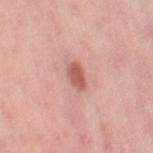Impression:
No biopsy was performed on this lesion — it was imaged during a full skin examination and was not determined to be concerning.
Context:
The lesion-visualizer software estimated a lesion area of about 4.5 mm², a shape eccentricity near 0.75, and two-axis asymmetry of about 0.15. And it measured a border-irregularity index near 1.5/10. And it measured a nevus-likeness score of about 90/100 and a lesion-detection confidence of about 100/100. Measured at roughly 3 mm in maximum diameter. A 15 mm close-up extracted from a 3D total-body photography capture. The lesion is on the right thigh. A female patient, aged around 50. The tile uses white-light illumination.